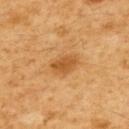Recorded during total-body skin imaging; not selected for excision or biopsy.
The lesion is located on the upper back.
Measured at roughly 3.5 mm in maximum diameter.
Captured under cross-polarized illumination.
A male subject, aged around 60.
A lesion tile, about 15 mm wide, cut from a 3D total-body photograph.